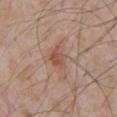Impression:
Part of a total-body skin-imaging series; this lesion was reviewed on a skin check and was not flagged for biopsy.
Context:
About 2.5 mm across. A 15 mm crop from a total-body photograph taken for skin-cancer surveillance. Located on the chest. Captured under white-light illumination. Automated tile analysis of the lesion measured an area of roughly 4.5 mm², an outline eccentricity of about 0.6 (0 = round, 1 = elongated), and a symmetry-axis asymmetry near 0.3. The analysis additionally found a border-irregularity rating of about 2.5/10 and a color-variation rating of about 3/10. A male subject, about 65 years old.